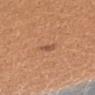<tbp_lesion>
<biopsy_status>not biopsied; imaged during a skin examination</biopsy_status>
<site>right forearm</site>
<image>
  <source>total-body photography crop</source>
  <field_of_view_mm>15</field_of_view_mm>
</image>
<lesion_size>
  <long_diameter_mm_approx>3.0</long_diameter_mm_approx>
</lesion_size>
<patient>
  <sex>female</sex>
  <age_approx>25</age_approx>
</patient>
<lighting>white-light</lighting>
</tbp_lesion>